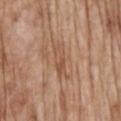notes: imaged on a skin check; not biopsied | image source: 15 mm crop, total-body photography | lesion diameter: about 4.5 mm | lighting: white-light illumination | subject: male, about 70 years old | body site: the mid back.